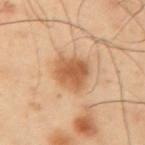The lesion was photographed on a routine skin check and not biopsied; there is no pathology result.
Imaged with cross-polarized lighting.
A male patient, aged around 40.
The lesion is on the arm.
Cropped from a total-body skin-imaging series; the visible field is about 15 mm.
Approximately 3.5 mm at its widest.
The total-body-photography lesion software estimated an eccentricity of roughly 0.4. The software also gave an average lesion color of about L≈58 a*≈23 b*≈38 (CIELAB), about 12 CIELAB-L* units darker than the surrounding skin, and a normalized border contrast of about 8.5. And it measured border irregularity of about 2 on a 0–10 scale, a within-lesion color-variation index near 3.5/10, and radial color variation of about 1. And it measured a nevus-likeness score of about 90/100.